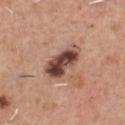<tbp_lesion>
<site>chest</site>
<patient>
  <sex>male</sex>
  <age_approx>45</age_approx>
</patient>
<automated_metrics>
  <area_mm2_approx>11.0</area_mm2_approx>
  <shape_asymmetry>0.25</shape_asymmetry>
  <vs_skin_darker_L>18.0</vs_skin_darker_L>
  <vs_skin_contrast_norm>13.0</vs_skin_contrast_norm>
  <color_variation_0_10>7.5</color_variation_0_10>
  <peripheral_color_asymmetry>3.0</peripheral_color_asymmetry>
  <nevus_likeness_0_100>30</nevus_likeness_0_100>
  <lesion_detection_confidence_0_100>100</lesion_detection_confidence_0_100>
</automated_metrics>
<lesion_size>
  <long_diameter_mm_approx>5.0</long_diameter_mm_approx>
</lesion_size>
<lighting>white-light</lighting>
<image>
  <source>total-body photography crop</source>
  <field_of_view_mm>15</field_of_view_mm>
</image>
</tbp_lesion>